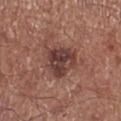No biopsy was performed on this lesion — it was imaged during a full skin examination and was not determined to be concerning.
About 4 mm across.
The lesion is on the right lower leg.
A lesion tile, about 15 mm wide, cut from a 3D total-body photograph.
The patient is a male approximately 70 years of age.
The tile uses white-light illumination.